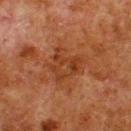notes: no biopsy performed (imaged during a skin exam) | lesion size: about 4.5 mm | anatomic site: the upper back | automated lesion analysis: a footprint of about 12 mm², an outline eccentricity of about 0.65 (0 = round, 1 = elongated), and two-axis asymmetry of about 0.45; a mean CIELAB color near L≈31 a*≈22 b*≈30, roughly 6 lightness units darker than nearby skin, and a normalized lesion–skin contrast near 6; a lesion-detection confidence of about 100/100 | acquisition: ~15 mm crop, total-body skin-cancer survey | patient: male, approximately 80 years of age.